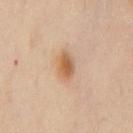Recorded during total-body skin imaging; not selected for excision or biopsy. This is a cross-polarized tile. About 3 mm across. A male patient aged 43 to 47. The lesion is located on the back. Automated tile analysis of the lesion measured an area of roughly 5.5 mm², a shape eccentricity near 0.75, and two-axis asymmetry of about 0.15. It also reported a mean CIELAB color near L≈57 a*≈19 b*≈35, about 11 CIELAB-L* units darker than the surrounding skin, and a normalized lesion–skin contrast near 8.5. And it measured a nevus-likeness score of about 95/100 and a lesion-detection confidence of about 100/100. A close-up tile cropped from a whole-body skin photograph, about 15 mm across.